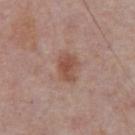This lesion was catalogued during total-body skin photography and was not selected for biopsy.
The recorded lesion diameter is about 3.5 mm.
The tile uses white-light illumination.
From the chest.
A close-up tile cropped from a whole-body skin photograph, about 15 mm across.
A male subject, aged approximately 75.
An algorithmic analysis of the crop reported an automated nevus-likeness rating near 55 out of 100 and lesion-presence confidence of about 100/100.